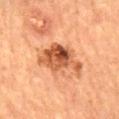Q: Patient demographics?
A: female, roughly 70 years of age
Q: How was this image acquired?
A: total-body-photography crop, ~15 mm field of view
Q: Lesion size?
A: about 6 mm
Q: What is the anatomic site?
A: the mid back
Q: What did automated image analysis measure?
A: an eccentricity of roughly 0.75 and a symmetry-axis asymmetry near 0.4; a border-irregularity index near 5/10, a within-lesion color-variation index near 9/10, and radial color variation of about 3; a classifier nevus-likeness of about 25/100 and a lesion-detection confidence of about 100/100